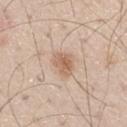workup: catalogued during a skin exam; not biopsied
location: the leg
size: about 3 mm
patient: male, aged around 60
image: total-body-photography crop, ~15 mm field of view
tile lighting: white-light